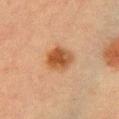This lesion was catalogued during total-body skin photography and was not selected for biopsy.
The patient is a female aged 53 to 57.
Captured under cross-polarized illumination.
Cropped from a whole-body photographic skin survey; the tile spans about 15 mm.
Longest diameter approximately 3.5 mm.
On the front of the torso.